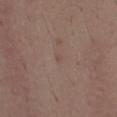notes — no biopsy performed (imaged during a skin exam) | automated lesion analysis — an area of roughly 1 mm², an eccentricity of roughly 0.6, and a shape-asymmetry score of about 0.2 (0 = symmetric); an average lesion color of about L≈48 a*≈16 b*≈22 (CIELAB) and a lesion–skin lightness drop of about 5; a border-irregularity rating of about 1.5/10 and a within-lesion color-variation index near 0/10 | location — the chest | tile lighting — white-light illumination | patient — male, approximately 40 years of age | imaging modality — ~15 mm tile from a whole-body skin photo.